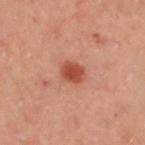Q: Was a biopsy performed?
A: imaged on a skin check; not biopsied
Q: How was this image acquired?
A: 15 mm crop, total-body photography
Q: How large is the lesion?
A: about 3 mm
Q: Patient demographics?
A: female, in their 40s
Q: Lesion location?
A: the upper back